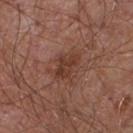| key | value |
|---|---|
| workup | catalogued during a skin exam; not biopsied |
| subject | male, aged 53 to 57 |
| location | the chest |
| image | total-body-photography crop, ~15 mm field of view |
| lesion diameter | ~4 mm (longest diameter) |
| illumination | white-light |